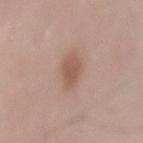No biopsy was performed on this lesion — it was imaged during a full skin examination and was not determined to be concerning.
A male subject aged 48 to 52.
About 3 mm across.
A 15 mm close-up tile from a total-body photography series done for melanoma screening.
From the lower back.